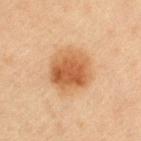Assessment: Imaged during a routine full-body skin examination; the lesion was not biopsied and no histopathology is available. Background: Cropped from a total-body skin-imaging series; the visible field is about 15 mm. Imaged with cross-polarized lighting. Measured at roughly 5 mm in maximum diameter. A female patient, approximately 45 years of age. The total-body-photography lesion software estimated an area of roughly 18 mm², an eccentricity of roughly 0.4, and a symmetry-axis asymmetry near 0.15. The software also gave a lesion color around L≈51 a*≈21 b*≈36 in CIELAB, a lesion–skin lightness drop of about 11, and a normalized lesion–skin contrast near 8.5. Located on the left upper arm.